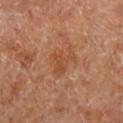Assessment: Captured during whole-body skin photography for melanoma surveillance; the lesion was not biopsied.